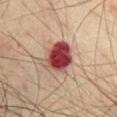Clinical impression:
Captured during whole-body skin photography for melanoma surveillance; the lesion was not biopsied.
Context:
This image is a 15 mm lesion crop taken from a total-body photograph. A male patient, aged approximately 75. On the chest. Longest diameter approximately 4.5 mm. This is a cross-polarized tile.